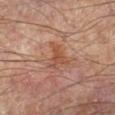<record>
<biopsy_status>not biopsied; imaged during a skin examination</biopsy_status>
<patient>
  <sex>male</sex>
  <age_approx>70</age_approx>
</patient>
<lighting>cross-polarized</lighting>
<automated_metrics>
  <area_mm2_approx>6.5</area_mm2_approx>
  <eccentricity>0.65</eccentricity>
  <shape_asymmetry>0.6</shape_asymmetry>
  <vs_skin_darker_L>7.0</vs_skin_darker_L>
  <vs_skin_contrast_norm>6.5</vs_skin_contrast_norm>
  <border_irregularity_0_10>6.5</border_irregularity_0_10>
  <peripheral_color_asymmetry>1.5</peripheral_color_asymmetry>
  <nevus_likeness_0_100>0</nevus_likeness_0_100>
</automated_metrics>
<site>left lower leg</site>
<image>
  <source>total-body photography crop</source>
  <field_of_view_mm>15</field_of_view_mm>
</image>
<lesion_size>
  <long_diameter_mm_approx>3.5</long_diameter_mm_approx>
</lesion_size>
</record>